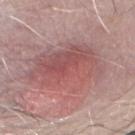| field | value |
|---|---|
| notes | imaged on a skin check; not biopsied |
| acquisition | ~15 mm tile from a whole-body skin photo |
| lesion diameter | about 8.5 mm |
| site | the front of the torso |
| image-analysis metrics | a mean CIELAB color near L≈53 a*≈24 b*≈21 and about 9 CIELAB-L* units darker than the surrounding skin; a border-irregularity index near 3.5/10, a color-variation rating of about 6/10, and a peripheral color-asymmetry measure near 1.5 |
| tile lighting | white-light illumination |
| patient | male, aged 78–82 |